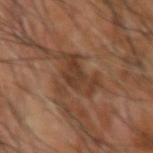workup=catalogued during a skin exam; not biopsied | patient=male, about 65 years old | acquisition=~15 mm crop, total-body skin-cancer survey | site=the right forearm.